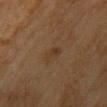follow-up=imaged on a skin check; not biopsied
lighting=cross-polarized
site=the chest
patient=female, in their mid- to late 50s
size=≈2.5 mm
acquisition=~15 mm tile from a whole-body skin photo
TBP lesion metrics=a lesion color around L≈31 a*≈15 b*≈27 in CIELAB and a normalized border contrast of about 5.5; a color-variation rating of about 1/10 and peripheral color asymmetry of about 0.5; a nevus-likeness score of about 45/100 and lesion-presence confidence of about 100/100